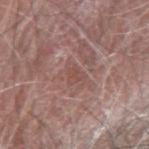biopsy status: no biopsy performed (imaged during a skin exam); patient: male, aged 83 or older; site: the right forearm; size: ~2.5 mm (longest diameter); illumination: white-light illumination; image source: total-body-photography crop, ~15 mm field of view.